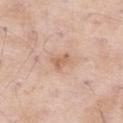biopsy status — total-body-photography surveillance lesion; no biopsy
illumination — white-light illumination
image — 15 mm crop, total-body photography
location — the left thigh
patient — male, in their mid- to late 50s